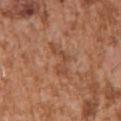follow-up — no biopsy performed (imaged during a skin exam)
subject — male, aged 43–47
body site — the left upper arm
image — total-body-photography crop, ~15 mm field of view